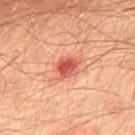{
  "biopsy_status": "not biopsied; imaged during a skin examination",
  "automated_metrics": {
    "area_mm2_approx": 5.5,
    "eccentricity": 0.5,
    "shape_asymmetry": 0.2,
    "cielab_L": 46,
    "cielab_a": 30,
    "cielab_b": 28,
    "vs_skin_darker_L": 12.0,
    "vs_skin_contrast_norm": 8.5,
    "border_irregularity_0_10": 2.0,
    "color_variation_0_10": 4.0,
    "nevus_likeness_0_100": 10,
    "lesion_detection_confidence_0_100": 100
  },
  "lesion_size": {
    "long_diameter_mm_approx": 2.5
  },
  "site": "chest",
  "patient": {
    "sex": "male",
    "age_approx": 75
  },
  "image": {
    "source": "total-body photography crop",
    "field_of_view_mm": 15
  }
}